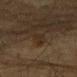  biopsy_status: not biopsied; imaged during a skin examination
  image:
    source: total-body photography crop
    field_of_view_mm: 15
  site: chest
  patient:
    sex: male
    age_approx: 65